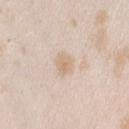biopsy_status: not biopsied; imaged during a skin examination
site: right upper arm
patient:
  sex: female
  age_approx: 25
image:
  source: total-body photography crop
  field_of_view_mm: 15